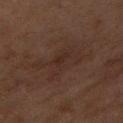A region of skin cropped from a whole-body photographic capture, roughly 15 mm wide. From the right forearm. Imaged with cross-polarized lighting. A female subject, in their 60s. Approximately 6.5 mm at its widest.